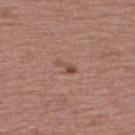Clinical impression:
Part of a total-body skin-imaging series; this lesion was reviewed on a skin check and was not flagged for biopsy.
Image and clinical context:
Imaged with white-light lighting. A male subject in their mid-50s. A 15 mm close-up tile from a total-body photography series done for melanoma screening. The recorded lesion diameter is about 2.5 mm. The lesion is on the upper back.